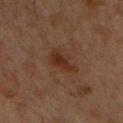This lesion was catalogued during total-body skin photography and was not selected for biopsy. Located on the upper back. A 15 mm close-up tile from a total-body photography series done for melanoma screening. Captured under cross-polarized illumination. About 3.5 mm across. A male subject approximately 65 years of age.